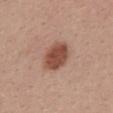Q: Was a biopsy performed?
A: imaged on a skin check; not biopsied
Q: What kind of image is this?
A: 15 mm crop, total-body photography
Q: Who is the patient?
A: female, aged approximately 30
Q: How was the tile lit?
A: white-light illumination
Q: How large is the lesion?
A: about 4 mm
Q: Lesion location?
A: the upper back
Q: Automated lesion metrics?
A: internal color variation of about 3 on a 0–10 scale and a peripheral color-asymmetry measure near 1